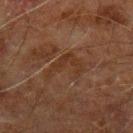The lesion was tiled from a total-body skin photograph and was not biopsied. This is a cross-polarized tile. The lesion is on the left upper arm. The lesion's longest dimension is about 4.5 mm. Cropped from a total-body skin-imaging series; the visible field is about 15 mm. Automated image analysis of the tile measured a lesion area of about 7.5 mm², a shape eccentricity near 0.75, and a shape-asymmetry score of about 0.7 (0 = symmetric). It also reported a mean CIELAB color near L≈25 a*≈15 b*≈23, a lesion–skin lightness drop of about 4, and a normalized lesion–skin contrast near 5.5. The software also gave a border-irregularity rating of about 9/10 and a within-lesion color-variation index near 1.5/10. And it measured an automated nevus-likeness rating near 0 out of 100. The subject is a male aged 58–62.